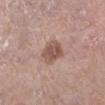Findings:
• biopsy status — catalogued during a skin exam; not biopsied
• body site — the left lower leg
• TBP lesion metrics — a within-lesion color-variation index near 3/10
• tile lighting — white-light
• subject — male, aged around 55
• image — 15 mm crop, total-body photography
• diameter — about 3.5 mm